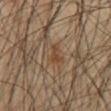Context:
A male patient approximately 60 years of age. A region of skin cropped from a whole-body photographic capture, roughly 15 mm wide. Located on the front of the torso.